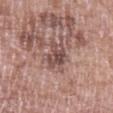Captured under white-light illumination. A 15 mm crop from a total-body photograph taken for skin-cancer surveillance. Approximately 4.5 mm at its widest. A female subject, approximately 70 years of age. The lesion-visualizer software estimated a border-irregularity rating of about 4.5/10 and a peripheral color-asymmetry measure near 2. The lesion is on the left upper arm.